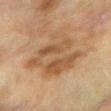Assessment: No biopsy was performed on this lesion — it was imaged during a full skin examination and was not determined to be concerning. Background: Cropped from a total-body skin-imaging series; the visible field is about 15 mm. The tile uses cross-polarized illumination. A female patient roughly 60 years of age. From the right forearm.